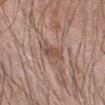workup=no biopsy performed (imaged during a skin exam); subject=male, aged 68 to 72; lesion size=about 3.5 mm; site=the left forearm; image source=~15 mm crop, total-body skin-cancer survey.